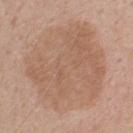Q: Was this lesion biopsied?
A: catalogued during a skin exam; not biopsied
Q: Patient demographics?
A: female, aged 53–57
Q: What is the imaging modality?
A: 15 mm crop, total-body photography
Q: Lesion location?
A: the back
Q: How large is the lesion?
A: about 10 mm